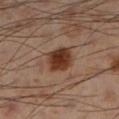notes: no biopsy performed (imaged during a skin exam)
image: ~15 mm crop, total-body skin-cancer survey
size: ~3.5 mm (longest diameter)
patient: male, approximately 55 years of age
illumination: cross-polarized
automated lesion analysis: an eccentricity of roughly 0.5 and a shape-asymmetry score of about 0.15 (0 = symmetric); a border-irregularity index near 1.5/10 and internal color variation of about 3.5 on a 0–10 scale
site: the leg